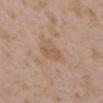Case summary:
– notes — total-body-photography surveillance lesion; no biopsy
– tile lighting — white-light illumination
– automated metrics — internal color variation of about 2 on a 0–10 scale and radial color variation of about 1; a detector confidence of about 100 out of 100 that the crop contains a lesion
– patient — female, roughly 35 years of age
– location — the left upper arm
– imaging modality — ~15 mm tile from a whole-body skin photo